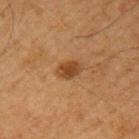Q: Is there a histopathology result?
A: total-body-photography surveillance lesion; no biopsy
Q: How was the tile lit?
A: cross-polarized illumination
Q: How was this image acquired?
A: ~15 mm crop, total-body skin-cancer survey
Q: How large is the lesion?
A: ≈3 mm
Q: Patient demographics?
A: male, aged approximately 65
Q: Where on the body is the lesion?
A: the left upper arm